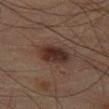Acquisition and patient details: Measured at roughly 4.5 mm in maximum diameter. Located on the left thigh. Captured under cross-polarized illumination. A male subject aged around 65. A region of skin cropped from a whole-body photographic capture, roughly 15 mm wide.